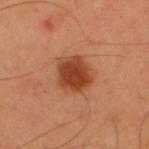Q: Was this lesion biopsied?
A: imaged on a skin check; not biopsied
Q: Who is the patient?
A: male, about 55 years old
Q: What kind of image is this?
A: total-body-photography crop, ~15 mm field of view
Q: What is the anatomic site?
A: the upper back
Q: Illumination type?
A: cross-polarized illumination
Q: What did automated image analysis measure?
A: a lesion color around L≈43 a*≈28 b*≈35 in CIELAB and a normalized border contrast of about 9.5; a color-variation rating of about 3.5/10
Q: Lesion size?
A: ~4 mm (longest diameter)